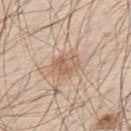Cropped from a total-body skin-imaging series; the visible field is about 15 mm. A male subject aged around 80. Captured under white-light illumination. Located on the back.